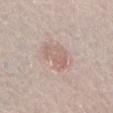Imaged during a routine full-body skin examination; the lesion was not biopsied and no histopathology is available.